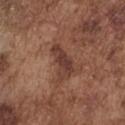Q: Is there a histopathology result?
A: imaged on a skin check; not biopsied
Q: What is the imaging modality?
A: total-body-photography crop, ~15 mm field of view
Q: Lesion size?
A: about 4.5 mm
Q: What did automated image analysis measure?
A: a footprint of about 7 mm² and a shape eccentricity near 0.9; a border-irregularity rating of about 5/10, a color-variation rating of about 2.5/10, and peripheral color asymmetry of about 0.5; an automated nevus-likeness rating near 5 out of 100 and a detector confidence of about 95 out of 100 that the crop contains a lesion
Q: Who is the patient?
A: male, roughly 75 years of age
Q: How was the tile lit?
A: white-light
Q: What is the anatomic site?
A: the chest